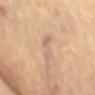workup=imaged on a skin check; not biopsied
site=the chest
lighting=cross-polarized illumination
size=≈4 mm
acquisition=~15 mm tile from a whole-body skin photo
patient=female, aged around 80